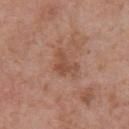notes: imaged on a skin check; not biopsied
size: ~3 mm (longest diameter)
illumination: white-light
body site: the chest
image: ~15 mm crop, total-body skin-cancer survey
patient: male, approximately 70 years of age